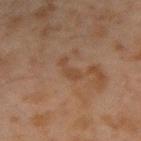{
  "site": "right forearm",
  "lesion_size": {
    "long_diameter_mm_approx": 3.5
  },
  "patient": {
    "sex": "male",
    "age_approx": 45
  },
  "image": {
    "source": "total-body photography crop",
    "field_of_view_mm": 15
  },
  "lighting": "cross-polarized"
}